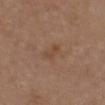| field | value |
|---|---|
| follow-up | no biopsy performed (imaged during a skin exam) |
| imaging modality | 15 mm crop, total-body photography |
| automated lesion analysis | a lesion color around L≈46 a*≈19 b*≈30 in CIELAB and a normalized border contrast of about 5; an automated nevus-likeness rating near 0 out of 100 |
| illumination | white-light illumination |
| subject | female, aged approximately 40 |
| anatomic site | the chest |